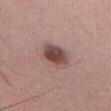workup: imaged on a skin check; not biopsied